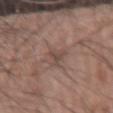Assessment: Recorded during total-body skin imaging; not selected for excision or biopsy. Clinical summary: Captured under white-light illumination. The subject is a male in their mid-70s. The recorded lesion diameter is about 3.5 mm. Cropped from a whole-body photographic skin survey; the tile spans about 15 mm. From the right forearm.